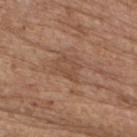| feature | finding |
|---|---|
| follow-up | catalogued during a skin exam; not biopsied |
| tile lighting | white-light illumination |
| diameter | ≈3 mm |
| subject | female, in their mid- to late 60s |
| anatomic site | the right upper arm |
| image | 15 mm crop, total-body photography |